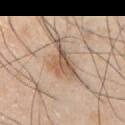Findings:
* workup · no biopsy performed (imaged during a skin exam)
* imaging modality · ~15 mm tile from a whole-body skin photo
* subject · male, aged approximately 30
* site · the chest